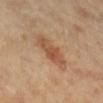{
  "biopsy_status": "not biopsied; imaged during a skin examination",
  "patient": {
    "sex": "female",
    "age_approx": 60
  },
  "image": {
    "source": "total-body photography crop",
    "field_of_view_mm": 15
  },
  "site": "right lower leg",
  "lighting": "cross-polarized",
  "lesion_size": {
    "long_diameter_mm_approx": 5.5
  },
  "automated_metrics": {
    "area_mm2_approx": 11.0,
    "eccentricity": 0.9,
    "shape_asymmetry": 0.15,
    "cielab_L": 54,
    "cielab_a": 21,
    "cielab_b": 34,
    "vs_skin_darker_L": 9.0,
    "vs_skin_contrast_norm": 6.5,
    "nevus_likeness_0_100": 60,
    "lesion_detection_confidence_0_100": 100
  }
}